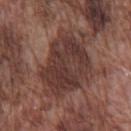No biopsy was performed on this lesion — it was imaged during a full skin examination and was not determined to be concerning.